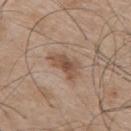biopsy status: total-body-photography surveillance lesion; no biopsy.